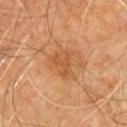<tbp_lesion>
<biopsy_status>not biopsied; imaged during a skin examination</biopsy_status>
<image>
  <source>total-body photography crop</source>
  <field_of_view_mm>15</field_of_view_mm>
</image>
<lesion_size>
  <long_diameter_mm_approx>3.0</long_diameter_mm_approx>
</lesion_size>
<lighting>cross-polarized</lighting>
<site>chest</site>
<patient>
  <sex>male</sex>
  <age_approx>60</age_approx>
</patient>
<automated_metrics>
  <nevus_likeness_0_100>0</nevus_likeness_0_100>
</automated_metrics>
</tbp_lesion>